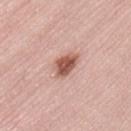Clinical impression:
Imaged during a routine full-body skin examination; the lesion was not biopsied and no histopathology is available.
Background:
An algorithmic analysis of the crop reported a classifier nevus-likeness of about 95/100. A female patient roughly 70 years of age. A 15 mm close-up tile from a total-body photography series done for melanoma screening. The tile uses white-light illumination. From the back. Longest diameter approximately 3.5 mm.